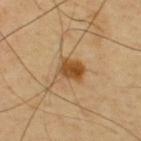A male patient aged 63 to 67.
On the upper back.
A region of skin cropped from a whole-body photographic capture, roughly 15 mm wide.
This is a cross-polarized tile.